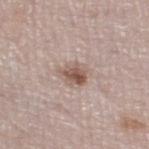{"biopsy_status": "not biopsied; imaged during a skin examination", "patient": {"sex": "male", "age_approx": 80}, "lesion_size": {"long_diameter_mm_approx": 3.0}, "automated_metrics": {"area_mm2_approx": 6.5, "eccentricity": 0.6, "shape_asymmetry": 0.2, "cielab_L": 55, "cielab_a": 16, "cielab_b": 24, "vs_skin_darker_L": 11.0, "vs_skin_contrast_norm": 8.0}, "image": {"source": "total-body photography crop", "field_of_view_mm": 15}, "lighting": "white-light", "site": "left lower leg"}